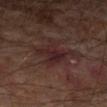| key | value |
|---|---|
| biopsy status | imaged on a skin check; not biopsied |
| patient | male, aged 63–67 |
| size | about 4 mm |
| automated lesion analysis | a border-irregularity index near 6.5/10 and a peripheral color-asymmetry measure near 1 |
| illumination | cross-polarized |
| body site | the left forearm |
| imaging modality | total-body-photography crop, ~15 mm field of view |